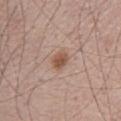<record>
<biopsy_status>not biopsied; imaged during a skin examination</biopsy_status>
<patient>
  <sex>male</sex>
  <age_approx>55</age_approx>
</patient>
<lighting>white-light</lighting>
<image>
  <source>total-body photography crop</source>
  <field_of_view_mm>15</field_of_view_mm>
</image>
</record>